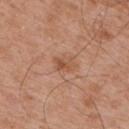Clinical impression:
Part of a total-body skin-imaging series; this lesion was reviewed on a skin check and was not flagged for biopsy.
Clinical summary:
The lesion is located on the right upper arm. The subject is a male about 55 years old. A roughly 15 mm field-of-view crop from a total-body skin photograph.